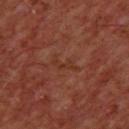{"biopsy_status": "not biopsied; imaged during a skin examination", "patient": {"sex": "male", "age_approx": 60}, "automated_metrics": {"border_irregularity_0_10": 7.0, "peripheral_color_asymmetry": 0.0, "nevus_likeness_0_100": 0}, "image": {"source": "total-body photography crop", "field_of_view_mm": 15}, "site": "chest", "lesion_size": {"long_diameter_mm_approx": 3.0}, "lighting": "cross-polarized"}